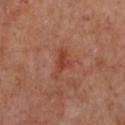This lesion was catalogued during total-body skin photography and was not selected for biopsy. The subject is a female aged 58 to 62. Imaged with cross-polarized lighting. The lesion is on the chest. Cropped from a total-body skin-imaging series; the visible field is about 15 mm. Longest diameter approximately 4 mm. The total-body-photography lesion software estimated a border-irregularity rating of about 5.5/10, a within-lesion color-variation index near 1.5/10, and radial color variation of about 0.5. The analysis additionally found a classifier nevus-likeness of about 0/100.